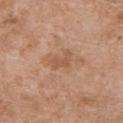Captured during whole-body skin photography for melanoma surveillance; the lesion was not biopsied.
The patient is a female aged around 60.
About 2.5 mm across.
Captured under white-light illumination.
A 15 mm close-up tile from a total-body photography series done for melanoma screening.
On the upper back.